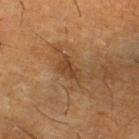Impression:
No biopsy was performed on this lesion — it was imaged during a full skin examination and was not determined to be concerning.
Acquisition and patient details:
The subject is a male aged 58 to 62. On the left lower leg. A close-up tile cropped from a whole-body skin photograph, about 15 mm across.